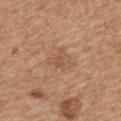Assessment: Recorded during total-body skin imaging; not selected for excision or biopsy. Background: The lesion's longest dimension is about 3 mm. An algorithmic analysis of the crop reported a border-irregularity rating of about 3/10, internal color variation of about 2 on a 0–10 scale, and a peripheral color-asymmetry measure near 0.5. Imaged with white-light lighting. A male patient, aged approximately 70. Located on the upper back. A 15 mm crop from a total-body photograph taken for skin-cancer surveillance.